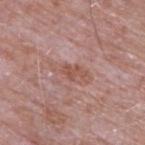This lesion was catalogued during total-body skin photography and was not selected for biopsy. Cropped from a whole-body photographic skin survey; the tile spans about 15 mm. A male patient roughly 65 years of age. The lesion is located on the upper back.